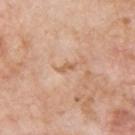Q: Lesion location?
A: the upper back
Q: What is the imaging modality?
A: ~15 mm tile from a whole-body skin photo
Q: Who is the patient?
A: male, aged approximately 60